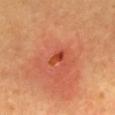A subject about 60 years old. Cropped from a total-body skin-imaging series; the visible field is about 15 mm. The lesion-visualizer software estimated a lesion area of about 2 mm², an outline eccentricity of about 0.85 (0 = round, 1 = elongated), and a symmetry-axis asymmetry near 0.3. The software also gave roughly 11 lightness units darker than nearby skin and a normalized lesion–skin contrast near 8. The analysis additionally found a border-irregularity index near 3/10, a color-variation rating of about 0/10, and peripheral color asymmetry of about 0. Captured under cross-polarized illumination. The lesion is located on the back. Measured at roughly 2.5 mm in maximum diameter. The biopsy diagnosis was an actinic keratosis, classified as an indeterminate (borderline) lesion.